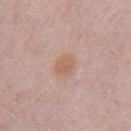follow-up: imaged on a skin check; not biopsied | diameter: ≈3 mm | body site: the left upper arm | TBP lesion metrics: a lesion area of about 5 mm², an eccentricity of roughly 0.6, and a shape-asymmetry score of about 0.2 (0 = symmetric); border irregularity of about 2 on a 0–10 scale, internal color variation of about 1.5 on a 0–10 scale, and peripheral color asymmetry of about 0.5; a classifier nevus-likeness of about 80/100 and a lesion-detection confidence of about 100/100 | subject: female, in their 30s | imaging modality: ~15 mm crop, total-body skin-cancer survey.